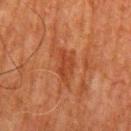No biopsy was performed on this lesion — it was imaged during a full skin examination and was not determined to be concerning. The subject is a male roughly 80 years of age. The lesion is located on the mid back. Approximately 4.5 mm at its widest. A 15 mm crop from a total-body photograph taken for skin-cancer surveillance.